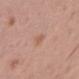No biopsy was performed on this lesion — it was imaged during a full skin examination and was not determined to be concerning.
Captured under white-light illumination.
Approximately 2.5 mm at its widest.
The subject is a male aged 38–42.
The lesion is on the back.
A 15 mm close-up tile from a total-body photography series done for melanoma screening.